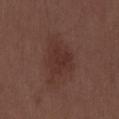workup: imaged on a skin check; not biopsied | image: ~15 mm crop, total-body skin-cancer survey | lighting: white-light illumination | diameter: ≈5.5 mm | body site: the back | patient: male, aged approximately 30.